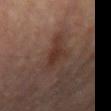Q: Was this lesion biopsied?
A: no biopsy performed (imaged during a skin exam)
Q: What are the patient's age and sex?
A: male, in their mid-80s or older
Q: What is the imaging modality?
A: 15 mm crop, total-body photography
Q: What is the anatomic site?
A: the left forearm